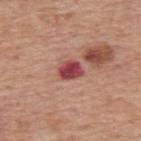  lighting: white-light
  lesion_size:
    long_diameter_mm_approx: 3.0
  patient:
    sex: male
    age_approx: 75
  image:
    source: total-body photography crop
    field_of_view_mm: 15
  site: upper back
  automated_metrics:
    area_mm2_approx: 5.5
    eccentricity: 0.65
    shape_asymmetry: 0.25
    border_irregularity_0_10: 2.0
    color_variation_0_10: 4.5
    peripheral_color_asymmetry: 1.5
    nevus_likeness_0_100: 0
    lesion_detection_confidence_0_100: 100